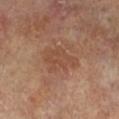{
  "biopsy_status": "not biopsied; imaged during a skin examination",
  "image": {
    "source": "total-body photography crop",
    "field_of_view_mm": 15
  },
  "lighting": "cross-polarized",
  "site": "right lower leg",
  "patient": {
    "sex": "female",
    "age_approx": 75
  }
}